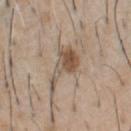Clinical impression:
Captured during whole-body skin photography for melanoma surveillance; the lesion was not biopsied.
Context:
A region of skin cropped from a whole-body photographic capture, roughly 15 mm wide. A male patient, aged 58 to 62. Imaged with white-light lighting. The lesion is located on the chest. An algorithmic analysis of the crop reported a footprint of about 8.5 mm² and a shape-asymmetry score of about 0.6 (0 = symmetric). It also reported an automated nevus-likeness rating near 80 out of 100.